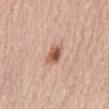{"biopsy_status": "not biopsied; imaged during a skin examination", "lesion_size": {"long_diameter_mm_approx": 2.5}, "patient": {"sex": "male", "age_approx": 65}, "image": {"source": "total-body photography crop", "field_of_view_mm": 15}, "site": "lower back", "automated_metrics": {"area_mm2_approx": 4.5, "eccentricity": 0.7, "shape_asymmetry": 0.2, "border_irregularity_0_10": 1.5, "peripheral_color_asymmetry": 2.0, "nevus_likeness_0_100": 95, "lesion_detection_confidence_0_100": 100}, "lighting": "white-light"}